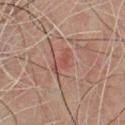Notes:
- notes · imaged on a skin check; not biopsied
- acquisition · ~15 mm tile from a whole-body skin photo
- body site · the chest
- lesion size · ~3.5 mm (longest diameter)
- TBP lesion metrics · an average lesion color of about L≈44 a*≈22 b*≈24 (CIELAB) and roughly 6 lightness units darker than nearby skin; a color-variation rating of about 2/10 and radial color variation of about 0.5
- tile lighting · cross-polarized
- subject · male, aged 58–62